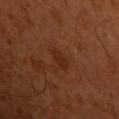Acquisition and patient details: The lesion is on the left upper arm. A close-up tile cropped from a whole-body skin photograph, about 15 mm across. The lesion's longest dimension is about 2.5 mm. A male subject, in their 50s. The total-body-photography lesion software estimated an average lesion color of about L≈27 a*≈23 b*≈31 (CIELAB), a lesion–skin lightness drop of about 6, and a normalized border contrast of about 6. And it measured a border-irregularity rating of about 3.5/10, a color-variation rating of about 0.5/10, and radial color variation of about 0. The software also gave a nevus-likeness score of about 35/100 and lesion-presence confidence of about 100/100. Captured under cross-polarized illumination.